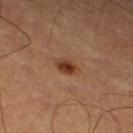image-analysis metrics: an area of roughly 4.5 mm², a shape eccentricity near 0.75, and a shape-asymmetry score of about 0.25 (0 = symmetric); lesion-presence confidence of about 100/100 | imaging modality: ~15 mm crop, total-body skin-cancer survey | site: the left thigh | illumination: cross-polarized illumination | lesion size: ~3 mm (longest diameter) | patient: male, aged 63 to 67.